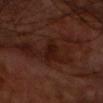A male subject in their 70s. A 15 mm close-up extracted from a 3D total-body photography capture. Approximately 3.5 mm at its widest. On the front of the torso.